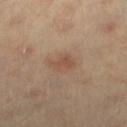Q: Is there a histopathology result?
A: catalogued during a skin exam; not biopsied
Q: Who is the patient?
A: about 60 years old
Q: How was the tile lit?
A: cross-polarized illumination
Q: How was this image acquired?
A: 15 mm crop, total-body photography
Q: What is the anatomic site?
A: the left lower leg
Q: How large is the lesion?
A: about 3 mm